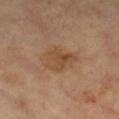Clinical impression:
Part of a total-body skin-imaging series; this lesion was reviewed on a skin check and was not flagged for biopsy.
Clinical summary:
A female subject about 70 years old. A roughly 15 mm field-of-view crop from a total-body skin photograph. The lesion is located on the right lower leg. Measured at roughly 4.5 mm in maximum diameter. This is a cross-polarized tile.